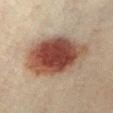On the abdomen. A female patient roughly 40 years of age. The recorded lesion diameter is about 9 mm. Captured under cross-polarized illumination. A lesion tile, about 15 mm wide, cut from a 3D total-body photograph.